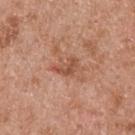Case summary:
• notes · imaged on a skin check; not biopsied
• tile lighting · white-light
• image-analysis metrics · an area of roughly 4 mm², an outline eccentricity of about 0.8 (0 = round, 1 = elongated), and a shape-asymmetry score of about 0.45 (0 = symmetric); a mean CIELAB color near L≈52 a*≈25 b*≈32, roughly 9 lightness units darker than nearby skin, and a normalized lesion–skin contrast near 6.5; lesion-presence confidence of about 100/100
• acquisition · 15 mm crop, total-body photography
• patient · male, aged 53–57
• lesion diameter · ~3 mm (longest diameter)
• location · the back